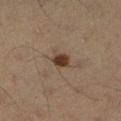notes = catalogued during a skin exam; not biopsied | size = ~2.5 mm (longest diameter) | site = the left lower leg | imaging modality = 15 mm crop, total-body photography | patient = male, aged 58–62.